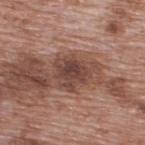| key | value |
|---|---|
| workup | imaged on a skin check; not biopsied |
| image | ~15 mm tile from a whole-body skin photo |
| TBP lesion metrics | an area of roughly 11 mm², a shape eccentricity near 0.5, and a symmetry-axis asymmetry near 0.3; a color-variation rating of about 4.5/10; a classifier nevus-likeness of about 0/100 and a detector confidence of about 100 out of 100 that the crop contains a lesion |
| subject | male, approximately 70 years of age |
| diameter | ~4 mm (longest diameter) |
| location | the upper back |